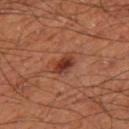follow-up = total-body-photography surveillance lesion; no biopsy
image source = 15 mm crop, total-body photography
patient = male, about 60 years old
anatomic site = the right thigh